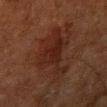follow-up — total-body-photography surveillance lesion; no biopsy | acquisition — ~15 mm crop, total-body skin-cancer survey | site — the right upper arm | lesion diameter — about 8 mm | subject — male, approximately 60 years of age | image-analysis metrics — a lesion color around L≈18 a*≈17 b*≈20 in CIELAB and a lesion-to-skin contrast of about 7 (normalized; higher = more distinct); a border-irregularity index near 7.5/10 and a color-variation rating of about 2.5/10.